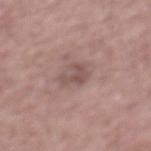Q: Was a biopsy performed?
A: catalogued during a skin exam; not biopsied
Q: Lesion location?
A: the mid back
Q: How was this image acquired?
A: 15 mm crop, total-body photography
Q: Patient demographics?
A: male, in their mid-50s